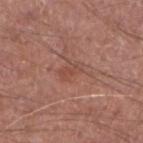biopsy status: no biopsy performed (imaged during a skin exam)
lighting: white-light illumination
patient: male, aged around 65
acquisition: total-body-photography crop, ~15 mm field of view
anatomic site: the right forearm